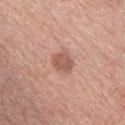follow-up: no biopsy performed (imaged during a skin exam)
diameter: about 3 mm
image: ~15 mm crop, total-body skin-cancer survey
site: the chest
subject: male, about 60 years old
tile lighting: white-light